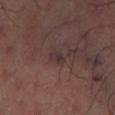Clinical impression: Part of a total-body skin-imaging series; this lesion was reviewed on a skin check and was not flagged for biopsy. Background: A roughly 15 mm field-of-view crop from a total-body skin photograph. The tile uses cross-polarized illumination. The recorded lesion diameter is about 2.5 mm. An algorithmic analysis of the crop reported an area of roughly 2.5 mm² and a symmetry-axis asymmetry near 0.35. And it measured a mean CIELAB color near L≈32 a*≈17 b*≈15 and about 6 CIELAB-L* units darker than the surrounding skin. The lesion is on the left thigh.